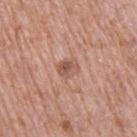biopsy status: imaged on a skin check; not biopsied
anatomic site: the right upper arm
patient: male, roughly 70 years of age
image source: 15 mm crop, total-body photography
tile lighting: white-light illumination
lesion diameter: ≈2.5 mm